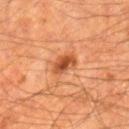No biopsy was performed on this lesion — it was imaged during a full skin examination and was not determined to be concerning.
Automated tile analysis of the lesion measured an automated nevus-likeness rating near 80 out of 100 and a lesion-detection confidence of about 100/100.
Measured at roughly 3 mm in maximum diameter.
A male subject, in their mid-60s.
Imaged with cross-polarized lighting.
A region of skin cropped from a whole-body photographic capture, roughly 15 mm wide.
On the right lower leg.